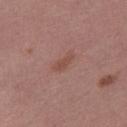Q: Is there a histopathology result?
A: no biopsy performed (imaged during a skin exam)
Q: How was the tile lit?
A: white-light illumination
Q: Lesion size?
A: ≈2.5 mm
Q: How was this image acquired?
A: 15 mm crop, total-body photography
Q: Who is the patient?
A: female, aged 48–52
Q: Where on the body is the lesion?
A: the right thigh